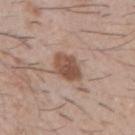subject — male, aged approximately 50 | anatomic site — the arm | automated metrics — a lesion color around L≈51 a*≈20 b*≈27 in CIELAB and a lesion–skin lightness drop of about 13; a border-irregularity index near 1.5/10, a within-lesion color-variation index near 4/10, and a peripheral color-asymmetry measure near 1.5; a nevus-likeness score of about 95/100 and a detector confidence of about 100 out of 100 that the crop contains a lesion | size — ~4 mm (longest diameter) | illumination — white-light | acquisition — ~15 mm tile from a whole-body skin photo.